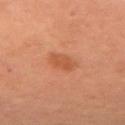From the arm.
This is a cross-polarized tile.
The patient is a female in their 60s.
A 15 mm crop from a total-body photograph taken for skin-cancer surveillance.